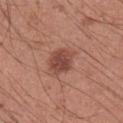| field | value |
|---|---|
| biopsy status | catalogued during a skin exam; not biopsied |
| patient | male, approximately 35 years of age |
| lighting | white-light illumination |
| lesion diameter | ≈3.5 mm |
| automated metrics | an area of roughly 7.5 mm², an outline eccentricity of about 0.55 (0 = round, 1 = elongated), and two-axis asymmetry of about 0.15 |
| site | the right upper arm |
| acquisition | total-body-photography crop, ~15 mm field of view |